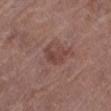The lesion was tiled from a total-body skin photograph and was not biopsied.
From the left lower leg.
Imaged with white-light lighting.
Longest diameter approximately 3.5 mm.
Cropped from a total-body skin-imaging series; the visible field is about 15 mm.
The subject is a female aged around 80.